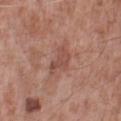Imaged during a routine full-body skin examination; the lesion was not biopsied and no histopathology is available. From the left lower leg. A lesion tile, about 15 mm wide, cut from a 3D total-body photograph. The subject is a male about 55 years old. The tile uses white-light illumination.